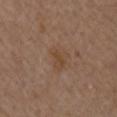This lesion was catalogued during total-body skin photography and was not selected for biopsy.
The subject is a male in their mid- to late 70s.
Automated tile analysis of the lesion measured lesion-presence confidence of about 100/100.
The lesion is on the right upper arm.
A lesion tile, about 15 mm wide, cut from a 3D total-body photograph.
Captured under white-light illumination.